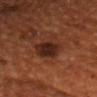Clinical impression: The lesion was photographed on a routine skin check and not biopsied; there is no pathology result. Acquisition and patient details: A 15 mm close-up tile from a total-body photography series done for melanoma screening. From the head or neck. The tile uses cross-polarized illumination. A male patient aged approximately 55. Automated image analysis of the tile measured an area of roughly 11 mm², a shape eccentricity near 0.45, and a shape-asymmetry score of about 0.25 (0 = symmetric). It also reported border irregularity of about 3.5 on a 0–10 scale, a within-lesion color-variation index near 4/10, and radial color variation of about 1.